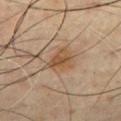Context: A male patient aged approximately 60. This image is a 15 mm lesion crop taken from a total-body photograph. Imaged with cross-polarized lighting. Longest diameter approximately 3.5 mm.